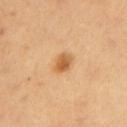Notes:
* follow-up — catalogued during a skin exam; not biopsied
* diameter — ~2.5 mm (longest diameter)
* tile lighting — cross-polarized illumination
* image — ~15 mm crop, total-body skin-cancer survey
* patient — female, in their mid-30s
* anatomic site — the arm